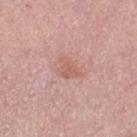| field | value |
|---|---|
| follow-up | total-body-photography surveillance lesion; no biopsy |
| patient | female, aged around 65 |
| tile lighting | white-light |
| image source | ~15 mm tile from a whole-body skin photo |
| location | the leg |